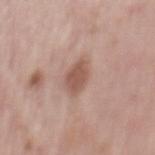| feature | finding |
|---|---|
| follow-up | no biopsy performed (imaged during a skin exam) |
| imaging modality | ~15 mm tile from a whole-body skin photo |
| tile lighting | white-light illumination |
| automated metrics | an outline eccentricity of about 0.75 (0 = round, 1 = elongated) and a symmetry-axis asymmetry near 0.3; a normalized lesion–skin contrast near 7.5; a border-irregularity index near 2.5/10, a within-lesion color-variation index near 1.5/10, and peripheral color asymmetry of about 0.5; a nevus-likeness score of about 80/100 and a detector confidence of about 100 out of 100 that the crop contains a lesion |
| patient | female, about 65 years old |
| site | the mid back |